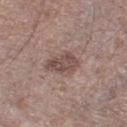Recorded during total-body skin imaging; not selected for excision or biopsy. This image is a 15 mm lesion crop taken from a total-body photograph. A male patient, aged approximately 70. Measured at roughly 4 mm in maximum diameter. The lesion-visualizer software estimated a normalized lesion–skin contrast near 7.5. The analysis additionally found a border-irregularity rating of about 2.5/10 and a color-variation rating of about 3.5/10. The software also gave a nevus-likeness score of about 5/100 and a lesion-detection confidence of about 100/100. The lesion is located on the left lower leg. Imaged with white-light lighting.